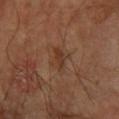Acquisition and patient details:
Longest diameter approximately 3 mm. A 15 mm crop from a total-body photograph taken for skin-cancer surveillance. The lesion-visualizer software estimated a lesion area of about 5 mm² and two-axis asymmetry of about 0.25. The software also gave an average lesion color of about L≈34 a*≈18 b*≈27 (CIELAB), about 6 CIELAB-L* units darker than the surrounding skin, and a normalized lesion–skin contrast near 6. And it measured a color-variation rating of about 2.5/10 and radial color variation of about 1. A male patient, about 70 years old. On the left forearm.